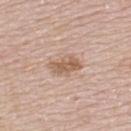Imaged during a routine full-body skin examination; the lesion was not biopsied and no histopathology is available.
The patient is a male roughly 70 years of age.
A 15 mm crop from a total-body photograph taken for skin-cancer surveillance.
The recorded lesion diameter is about 4 mm.
On the upper back.
An algorithmic analysis of the crop reported an average lesion color of about L≈60 a*≈18 b*≈30 (CIELAB), a lesion–skin lightness drop of about 10, and a normalized lesion–skin contrast near 7.5. It also reported border irregularity of about 3 on a 0–10 scale and a color-variation rating of about 3.5/10.
This is a white-light tile.